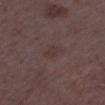{
  "biopsy_status": "not biopsied; imaged during a skin examination",
  "site": "right thigh",
  "image": {
    "source": "total-body photography crop",
    "field_of_view_mm": 15
  },
  "lighting": "white-light",
  "patient": {
    "sex": "female",
    "age_approx": 35
  }
}